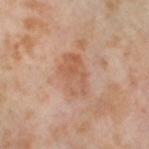| feature | finding |
|---|---|
| notes | catalogued during a skin exam; not biopsied |
| site | the left thigh |
| patient | female, aged 53–57 |
| illumination | cross-polarized |
| acquisition | total-body-photography crop, ~15 mm field of view |
| lesion diameter | ≈5 mm |